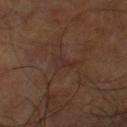Notes:
* notes · imaged on a skin check; not biopsied
* location · the right lower leg
* image-analysis metrics · a normalized border contrast of about 5
* image source · ~15 mm crop, total-body skin-cancer survey
* subject · male, aged around 60